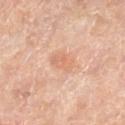Case summary:
• workup: no biopsy performed (imaged during a skin exam)
• TBP lesion metrics: a border-irregularity index near 3/10, a within-lesion color-variation index near 3/10, and peripheral color asymmetry of about 1
• body site: the right lower leg
• patient: male, aged approximately 65
• acquisition: total-body-photography crop, ~15 mm field of view
• lesion diameter: ~3 mm (longest diameter)
• tile lighting: cross-polarized